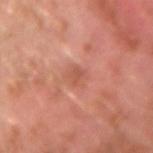Captured during whole-body skin photography for melanoma surveillance; the lesion was not biopsied.